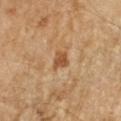{
  "biopsy_status": "not biopsied; imaged during a skin examination",
  "lighting": "cross-polarized",
  "image": {
    "source": "total-body photography crop",
    "field_of_view_mm": 15
  },
  "automated_metrics": {
    "cielab_L": 54,
    "cielab_a": 22,
    "cielab_b": 39,
    "vs_skin_darker_L": 11.0,
    "vs_skin_contrast_norm": 8.0,
    "border_irregularity_0_10": 3.0,
    "color_variation_0_10": 1.5,
    "nevus_likeness_0_100": 5
  },
  "lesion_size": {
    "long_diameter_mm_approx": 2.5
  },
  "patient": {
    "sex": "female",
    "age_approx": 70
  },
  "site": "arm"
}